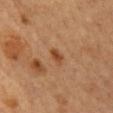The lesion was photographed on a routine skin check and not biopsied; there is no pathology result.
Imaged with cross-polarized lighting.
The lesion is located on the mid back.
The recorded lesion diameter is about 2.5 mm.
A roughly 15 mm field-of-view crop from a total-body skin photograph.
An algorithmic analysis of the crop reported a mean CIELAB color near L≈40 a*≈22 b*≈32 and roughly 9 lightness units darker than nearby skin. And it measured a border-irregularity index near 2.5/10, a color-variation rating of about 0/10, and radial color variation of about 0. It also reported an automated nevus-likeness rating near 95 out of 100 and lesion-presence confidence of about 100/100.
A female patient in their 60s.